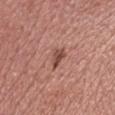No biopsy was performed on this lesion — it was imaged during a full skin examination and was not determined to be concerning. Captured under white-light illumination. Measured at roughly 3.5 mm in maximum diameter. A roughly 15 mm field-of-view crop from a total-body skin photograph. A male patient aged 28–32. The lesion-visualizer software estimated an area of roughly 3.5 mm² and a symmetry-axis asymmetry near 0.4. The software also gave a lesion color around L≈48 a*≈24 b*≈25 in CIELAB, a lesion–skin lightness drop of about 11, and a normalized lesion–skin contrast near 8. The software also gave a border-irregularity rating of about 4/10, a within-lesion color-variation index near 2/10, and a peripheral color-asymmetry measure near 0.5.